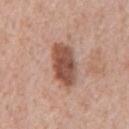Imaged during a routine full-body skin examination; the lesion was not biopsied and no histopathology is available. A region of skin cropped from a whole-body photographic capture, roughly 15 mm wide. From the chest. The patient is a male about 60 years old. Imaged with white-light lighting.